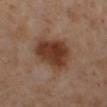Q: Who is the patient?
A: female, in their mid- to late 50s
Q: What is the imaging modality?
A: total-body-photography crop, ~15 mm field of view
Q: What is the anatomic site?
A: the left lower leg